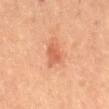Q: Was this lesion biopsied?
A: no biopsy performed (imaged during a skin exam)
Q: Who is the patient?
A: female, aged approximately 60
Q: How large is the lesion?
A: ~3 mm (longest diameter)
Q: What kind of image is this?
A: ~15 mm tile from a whole-body skin photo
Q: Where on the body is the lesion?
A: the mid back